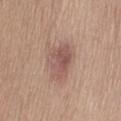automated metrics: a lesion area of about 10 mm², an outline eccentricity of about 0.65 (0 = round, 1 = elongated), and two-axis asymmetry of about 0.25; a mean CIELAB color near L≈54 a*≈20 b*≈24, a lesion–skin lightness drop of about 9, and a normalized lesion–skin contrast near 7; an automated nevus-likeness rating near 45 out of 100
location: the abdomen
size: ≈4.5 mm
imaging modality: total-body-photography crop, ~15 mm field of view
subject: female, in their mid-60s